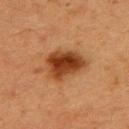Clinical impression:
No biopsy was performed on this lesion — it was imaged during a full skin examination and was not determined to be concerning.
Clinical summary:
A female patient, approximately 40 years of age. The tile uses cross-polarized illumination. A lesion tile, about 15 mm wide, cut from a 3D total-body photograph. Measured at roughly 5 mm in maximum diameter. The lesion is located on the back.